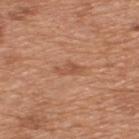Q: Is there a histopathology result?
A: no biopsy performed (imaged during a skin exam)
Q: What kind of image is this?
A: 15 mm crop, total-body photography
Q: Illumination type?
A: white-light
Q: Lesion location?
A: the upper back
Q: What are the patient's age and sex?
A: male, aged approximately 65
Q: Automated lesion metrics?
A: a footprint of about 3 mm², an eccentricity of roughly 0.9, and a shape-asymmetry score of about 0.45 (0 = symmetric)